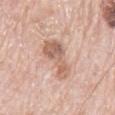{"biopsy_status": "not biopsied; imaged during a skin examination", "lesion_size": {"long_diameter_mm_approx": 6.5}, "site": "mid back", "image": {"source": "total-body photography crop", "field_of_view_mm": 15}, "lighting": "white-light", "patient": {"sex": "male", "age_approx": 80}}